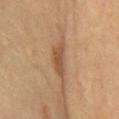patient = male, aged approximately 70
acquisition = total-body-photography crop, ~15 mm field of view
lesion diameter = ~5 mm (longest diameter)
TBP lesion metrics = an average lesion color of about L≈50 a*≈19 b*≈33 (CIELAB) and a normalized lesion–skin contrast near 7.5; an automated nevus-likeness rating near 35 out of 100 and a detector confidence of about 95 out of 100 that the crop contains a lesion
location = the chest